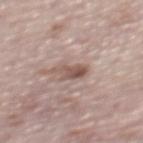Q: Is there a histopathology result?
A: no biopsy performed (imaged during a skin exam)